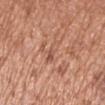Imaged during a routine full-body skin examination; the lesion was not biopsied and no histopathology is available. The lesion is located on the arm. The subject is a male in their mid- to late 70s. A lesion tile, about 15 mm wide, cut from a 3D total-body photograph.